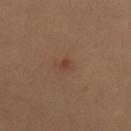follow-up: total-body-photography surveillance lesion; no biopsy
body site: the abdomen
size: about 1.5 mm
patient: female, aged around 30
automated metrics: an average lesion color of about L≈40 a*≈20 b*≈29 (CIELAB) and a lesion-to-skin contrast of about 6 (normalized; higher = more distinct); a border-irregularity rating of about 2/10; a nevus-likeness score of about 15/100
image: ~15 mm crop, total-body skin-cancer survey